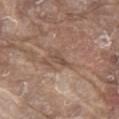subject = male, aged 78–82 | body site = the front of the torso | image = ~15 mm crop, total-body skin-cancer survey | TBP lesion metrics = an area of roughly 3 mm², an eccentricity of roughly 0.9, and a symmetry-axis asymmetry near 0.35; a lesion color around L≈49 a*≈17 b*≈26 in CIELAB, about 8 CIELAB-L* units darker than the surrounding skin, and a normalized lesion–skin contrast near 6 | illumination = white-light illumination.